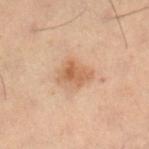Assessment:
Part of a total-body skin-imaging series; this lesion was reviewed on a skin check and was not flagged for biopsy.
Clinical summary:
The lesion's longest dimension is about 4 mm. A male patient, aged approximately 55. Cropped from a total-body skin-imaging series; the visible field is about 15 mm. The tile uses cross-polarized illumination. From the right lower leg.